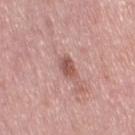This lesion was catalogued during total-body skin photography and was not selected for biopsy. The tile uses white-light illumination. From the right thigh. Measured at roughly 2.5 mm in maximum diameter. A 15 mm close-up extracted from a 3D total-body photography capture. A female patient aged 48–52.